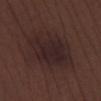Impression: Captured during whole-body skin photography for melanoma surveillance; the lesion was not biopsied. Image and clinical context: The recorded lesion diameter is about 7.5 mm. Cropped from a whole-body photographic skin survey; the tile spans about 15 mm. The lesion is located on the left lower leg. The tile uses white-light illumination. The patient is a male about 70 years old.